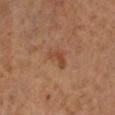follow-up = no biopsy performed (imaged during a skin exam)
TBP lesion metrics = a border-irregularity index near 5/10, a color-variation rating of about 2/10, and peripheral color asymmetry of about 0.5
location = the left lower leg
diameter = ≈3 mm
illumination = cross-polarized illumination
imaging modality = 15 mm crop, total-body photography
subject = male, about 60 years old